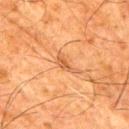Part of a total-body skin-imaging series; this lesion was reviewed on a skin check and was not flagged for biopsy.
The recorded lesion diameter is about 3 mm.
Cropped from a whole-body photographic skin survey; the tile spans about 15 mm.
A male subject aged approximately 80.
Located on the right upper arm.